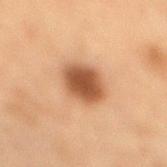Clinical impression: Captured during whole-body skin photography for melanoma surveillance; the lesion was not biopsied. Acquisition and patient details: The tile uses cross-polarized illumination. Located on the right lower leg. A female subject in their mid- to late 50s. Measured at roughly 4 mm in maximum diameter. Cropped from a whole-body photographic skin survey; the tile spans about 15 mm.